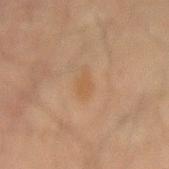This lesion was catalogued during total-body skin photography and was not selected for biopsy. A male subject, aged 43 to 47. The lesion is located on the mid back. This image is a 15 mm lesion crop taken from a total-body photograph.